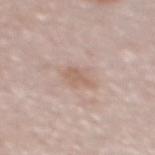Assessment: The lesion was tiled from a total-body skin photograph and was not biopsied. Clinical summary: A female subject about 50 years old. A 15 mm close-up extracted from a 3D total-body photography capture. About 2.5 mm across. The total-body-photography lesion software estimated a mean CIELAB color near L≈61 a*≈17 b*≈26 and a normalized lesion–skin contrast near 5.5. Imaged with white-light lighting. From the back.